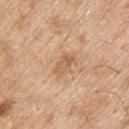anatomic site: the upper back
subject: male, aged 68 to 72
image-analysis metrics: an area of roughly 5 mm², an eccentricity of roughly 0.8, and a shape-asymmetry score of about 0.3 (0 = symmetric); an average lesion color of about L≈61 a*≈20 b*≈35 (CIELAB), about 8 CIELAB-L* units darker than the surrounding skin, and a normalized border contrast of about 5.5; a border-irregularity rating of about 3/10 and a peripheral color-asymmetry measure near 1; a nevus-likeness score of about 0/100 and a lesion-detection confidence of about 100/100
lighting: white-light
size: ≈3 mm
image: ~15 mm tile from a whole-body skin photo